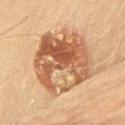The lesion was tiled from a total-body skin photograph and was not biopsied.
The tile uses cross-polarized illumination.
A 15 mm crop from a total-body photograph taken for skin-cancer surveillance.
A male subject in their 70s.
The lesion is on the upper back.
The recorded lesion diameter is about 7.5 mm.
The total-body-photography lesion software estimated a lesion–skin lightness drop of about 11 and a normalized border contrast of about 8.5. The software also gave a classifier nevus-likeness of about 45/100 and a lesion-detection confidence of about 100/100.